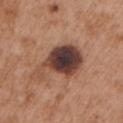follow-up: catalogued during a skin exam; not biopsied | lighting: white-light illumination | location: the left upper arm | lesion diameter: ~7 mm (longest diameter) | subject: male, aged around 55 | image: ~15 mm crop, total-body skin-cancer survey.